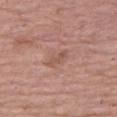The lesion was photographed on a routine skin check and not biopsied; there is no pathology result. Approximately 3 mm at its widest. A close-up tile cropped from a whole-body skin photograph, about 15 mm across. The tile uses white-light illumination. A female subject aged approximately 65. Located on the right thigh.